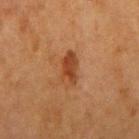Imaged during a routine full-body skin examination; the lesion was not biopsied and no histopathology is available.
The subject is a female in their mid-50s.
Automated image analysis of the tile measured a footprint of about 7 mm², an eccentricity of roughly 0.85, and a shape-asymmetry score of about 0.15 (0 = symmetric). The software also gave a lesion color around L≈36 a*≈22 b*≈31 in CIELAB, a lesion–skin lightness drop of about 8, and a normalized border contrast of about 7.5. The analysis additionally found a within-lesion color-variation index near 4/10 and radial color variation of about 1.5.
A 15 mm crop from a total-body photograph taken for skin-cancer surveillance.
Longest diameter approximately 4 mm.
The lesion is located on the right upper arm.